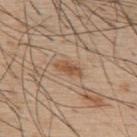Captured during whole-body skin photography for melanoma surveillance; the lesion was not biopsied. The tile uses white-light illumination. The lesion is on the upper back. Approximately 3.5 mm at its widest. A close-up tile cropped from a whole-body skin photograph, about 15 mm across. The patient is a male aged 53–57.